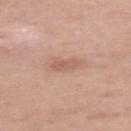| field | value |
|---|---|
| workup | total-body-photography surveillance lesion; no biopsy |
| body site | the upper back |
| patient | female, roughly 35 years of age |
| lesion diameter | about 2.5 mm |
| imaging modality | 15 mm crop, total-body photography |
| TBP lesion metrics | an area of roughly 3.5 mm² and a shape-asymmetry score of about 0.2 (0 = symmetric); a border-irregularity rating of about 2.5/10 and a color-variation rating of about 1.5/10; a classifier nevus-likeness of about 0/100 and a lesion-detection confidence of about 100/100 |
| lighting | white-light illumination |